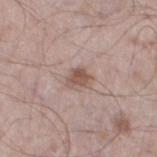Impression: Part of a total-body skin-imaging series; this lesion was reviewed on a skin check and was not flagged for biopsy. Clinical summary: Cropped from a whole-body photographic skin survey; the tile spans about 15 mm. The lesion is on the left thigh. The tile uses white-light illumination. The subject is a male aged 53–57. The lesion-visualizer software estimated an eccentricity of roughly 0.65 and a shape-asymmetry score of about 0.3 (0 = symmetric). The software also gave a mean CIELAB color near L≈53 a*≈18 b*≈24, a lesion–skin lightness drop of about 11, and a normalized border contrast of about 7.5. And it measured a border-irregularity index near 2.5/10, a within-lesion color-variation index near 3/10, and peripheral color asymmetry of about 1.